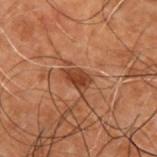Findings:
– subject · male, roughly 50 years of age
– automated lesion analysis · a border-irregularity rating of about 2.5/10, a within-lesion color-variation index near 2/10, and a peripheral color-asymmetry measure near 0.5
– lesion size · ≈3 mm
– acquisition · total-body-photography crop, ~15 mm field of view
– location · the back